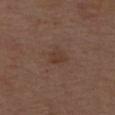{"patient": {"sex": "male", "age_approx": 70}, "lesion_size": {"long_diameter_mm_approx": 2.5}, "image": {"source": "total-body photography crop", "field_of_view_mm": 15}, "site": "upper back"}